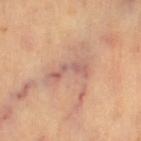site: left thigh
image:
  source: total-body photography crop
  field_of_view_mm: 15
patient:
  sex: female
  age_approx: 70
lesion_size:
  long_diameter_mm_approx: 4.5
lighting: cross-polarized